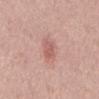Recorded during total-body skin imaging; not selected for excision or biopsy.
A close-up tile cropped from a whole-body skin photograph, about 15 mm across.
A male patient roughly 45 years of age.
On the back.